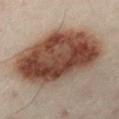Impression: Recorded during total-body skin imaging; not selected for excision or biopsy. Image and clinical context: Cropped from a whole-body photographic skin survey; the tile spans about 15 mm. The subject is a male aged 48 to 52. An algorithmic analysis of the crop reported a footprint of about 65 mm², a shape eccentricity near 0.85, and two-axis asymmetry of about 0.1. The software also gave a mean CIELAB color near L≈37 a*≈15 b*≈22, about 15 CIELAB-L* units darker than the surrounding skin, and a normalized border contrast of about 12.5. The tile uses cross-polarized illumination. The lesion is on the left lower leg.